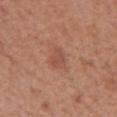notes: catalogued during a skin exam; not biopsied | image: total-body-photography crop, ~15 mm field of view | anatomic site: the abdomen | subject: male, roughly 65 years of age.